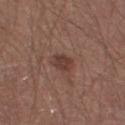workup = total-body-photography surveillance lesion; no biopsy.